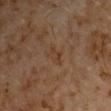Clinical impression:
This lesion was catalogued during total-body skin photography and was not selected for biopsy.
Context:
Automated tile analysis of the lesion measured a lesion area of about 3.5 mm², an eccentricity of roughly 0.85, and two-axis asymmetry of about 0.4. It also reported a lesion color around L≈35 a*≈16 b*≈28 in CIELAB, a lesion–skin lightness drop of about 4, and a normalized border contrast of about 5. It also reported an automated nevus-likeness rating near 0 out of 100 and a lesion-detection confidence of about 100/100. A male subject, aged around 60. Measured at roughly 3 mm in maximum diameter. The lesion is located on the left upper arm. A close-up tile cropped from a whole-body skin photograph, about 15 mm across.